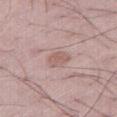Recorded during total-body skin imaging; not selected for excision or biopsy. Located on the leg. Captured under white-light illumination. An algorithmic analysis of the crop reported a lesion area of about 4.5 mm². The software also gave a lesion color around L≈58 a*≈19 b*≈22 in CIELAB and a lesion-to-skin contrast of about 5.5 (normalized; higher = more distinct). It also reported border irregularity of about 2.5 on a 0–10 scale, a color-variation rating of about 1.5/10, and peripheral color asymmetry of about 0.5. A roughly 15 mm field-of-view crop from a total-body skin photograph. The patient is a male aged 48 to 52. The recorded lesion diameter is about 2.5 mm.